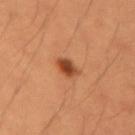The lesion's longest dimension is about 2.5 mm. A female subject in their 40s. Captured under cross-polarized illumination. The lesion is located on the right upper arm. A roughly 15 mm field-of-view crop from a total-body skin photograph.